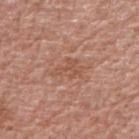biopsy_status: not biopsied; imaged during a skin examination
patient:
  sex: male
  age_approx: 70
lighting: white-light
site: right upper arm
automated_metrics:
  area_mm2_approx: 5.0
  eccentricity: 0.75
  shape_asymmetry: 0.45
  cielab_L: 53
  cielab_a: 23
  cielab_b: 30
  vs_skin_darker_L: 7.0
  vs_skin_contrast_norm: 5.0
image:
  source: total-body photography crop
  field_of_view_mm: 15